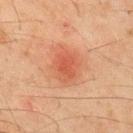Imaged during a routine full-body skin examination; the lesion was not biopsied and no histopathology is available. A close-up tile cropped from a whole-body skin photograph, about 15 mm across. From the upper back. Approximately 3.5 mm at its widest. The patient is a male in their mid- to late 40s.